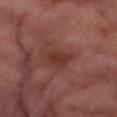Recorded during total-body skin imaging; not selected for excision or biopsy.
This is a cross-polarized tile.
The recorded lesion diameter is about 3.5 mm.
A female patient in their mid- to late 50s.
The lesion is on the right thigh.
Automated image analysis of the tile measured radial color variation of about 0.5. The analysis additionally found a lesion-detection confidence of about 90/100.
A 15 mm crop from a total-body photograph taken for skin-cancer surveillance.